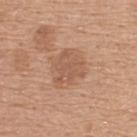| key | value |
|---|---|
| follow-up | no biopsy performed (imaged during a skin exam) |
| tile lighting | white-light illumination |
| lesion size | ~3.5 mm (longest diameter) |
| image-analysis metrics | a footprint of about 8.5 mm² and an eccentricity of roughly 0.3; about 8 CIELAB-L* units darker than the surrounding skin and a normalized lesion–skin contrast near 5.5; a border-irregularity rating of about 4.5/10, a color-variation rating of about 1.5/10, and radial color variation of about 0.5; a classifier nevus-likeness of about 0/100 |
| image | ~15 mm crop, total-body skin-cancer survey |
| site | the upper back |
| patient | female, aged 58 to 62 |